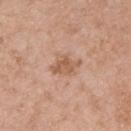The lesion was tiled from a total-body skin photograph and was not biopsied.
The lesion is on the left upper arm.
The tile uses white-light illumination.
A 15 mm close-up extracted from a 3D total-body photography capture.
A male subject roughly 80 years of age.
The lesion's longest dimension is about 4 mm.